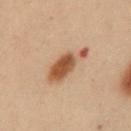Recorded during total-body skin imaging; not selected for excision or biopsy.
A 15 mm crop from a total-body photograph taken for skin-cancer surveillance.
Imaged with cross-polarized lighting.
About 6 mm across.
The subject is a female approximately 30 years of age.
On the mid back.